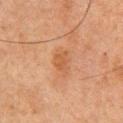biopsy status: no biopsy performed (imaged during a skin exam) | patient: male, about 75 years old | automated metrics: an area of roughly 4 mm², an outline eccentricity of about 0.75 (0 = round, 1 = elongated), and a shape-asymmetry score of about 0.25 (0 = symmetric); a classifier nevus-likeness of about 5/100 | size: ~2.5 mm (longest diameter) | image source: total-body-photography crop, ~15 mm field of view | anatomic site: the front of the torso | lighting: cross-polarized illumination.